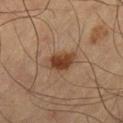Assessment:
This lesion was catalogued during total-body skin photography and was not selected for biopsy.
Context:
A roughly 15 mm field-of-view crop from a total-body skin photograph. An algorithmic analysis of the crop reported a lesion area of about 7 mm², an outline eccentricity of about 0.7 (0 = round, 1 = elongated), and a shape-asymmetry score of about 0.2 (0 = symmetric). And it measured border irregularity of about 2 on a 0–10 scale and radial color variation of about 1. The lesion is on the right thigh. A male patient in their mid-60s.